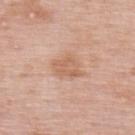Q: Was this lesion biopsied?
A: total-body-photography surveillance lesion; no biopsy
Q: How was this image acquired?
A: ~15 mm tile from a whole-body skin photo
Q: Who is the patient?
A: female, in their 50s
Q: How large is the lesion?
A: ~3.5 mm (longest diameter)
Q: Automated lesion metrics?
A: an outline eccentricity of about 0.6 (0 = round, 1 = elongated) and a shape-asymmetry score of about 0.3 (0 = symmetric); a border-irregularity index near 3/10, a within-lesion color-variation index near 2.5/10, and radial color variation of about 1
Q: What is the anatomic site?
A: the back